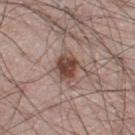Imaged during a routine full-body skin examination; the lesion was not biopsied and no histopathology is available. An algorithmic analysis of the crop reported a mean CIELAB color near L≈46 a*≈19 b*≈24 and roughly 14 lightness units darker than nearby skin. And it measured internal color variation of about 4 on a 0–10 scale and radial color variation of about 1.5. On the right leg. The subject is a male in their 70s. Imaged with white-light lighting. The recorded lesion diameter is about 3.5 mm. A 15 mm close-up tile from a total-body photography series done for melanoma screening.